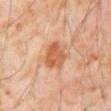{"biopsy_status": "not biopsied; imaged during a skin examination", "lesion_size": {"long_diameter_mm_approx": 4.0}, "image": {"source": "total-body photography crop", "field_of_view_mm": 15}, "automated_metrics": {"area_mm2_approx": 9.5, "eccentricity": 0.5, "shape_asymmetry": 0.2, "vs_skin_darker_L": 10.0, "vs_skin_contrast_norm": 8.0, "nevus_likeness_0_100": 75, "lesion_detection_confidence_0_100": 100}, "lighting": "cross-polarized", "site": "abdomen", "patient": {"sex": "male", "age_approx": 60}}